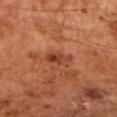notes: no biopsy performed (imaged during a skin exam)
lesion diameter: ~3 mm (longest diameter)
image-analysis metrics: an eccentricity of roughly 0.75 and a shape-asymmetry score of about 0.35 (0 = symmetric); a lesion color around L≈38 a*≈26 b*≈32 in CIELAB and a normalized lesion–skin contrast near 7.5
subject: male, aged 58–62
lighting: cross-polarized illumination
location: the right forearm
image source: ~15 mm crop, total-body skin-cancer survey